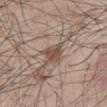Q: Was this lesion biopsied?
A: total-body-photography surveillance lesion; no biopsy
Q: What lighting was used for the tile?
A: white-light illumination
Q: Lesion size?
A: ~2.5 mm (longest diameter)
Q: What is the imaging modality?
A: total-body-photography crop, ~15 mm field of view
Q: Lesion location?
A: the abdomen
Q: What are the patient's age and sex?
A: male, aged around 60